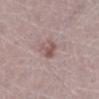{"biopsy_status": "not biopsied; imaged during a skin examination", "patient": {"sex": "female", "age_approx": 50}, "image": {"source": "total-body photography crop", "field_of_view_mm": 15}, "lesion_size": {"long_diameter_mm_approx": 2.5}, "site": "right lower leg", "lighting": "white-light"}